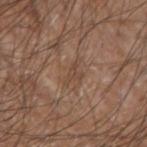Measured at roughly 3 mm in maximum diameter. From the left forearm. The total-body-photography lesion software estimated an area of roughly 3 mm², an outline eccentricity of about 0.85 (0 = round, 1 = elongated), and a symmetry-axis asymmetry near 0.6. The analysis additionally found a mean CIELAB color near L≈45 a*≈17 b*≈27, roughly 6 lightness units darker than nearby skin, and a normalized lesion–skin contrast near 5.5. The analysis additionally found a border-irregularity rating of about 7/10, a within-lesion color-variation index near 0/10, and radial color variation of about 0. The analysis additionally found a classifier nevus-likeness of about 0/100 and lesion-presence confidence of about 85/100. The tile uses white-light illumination. A 15 mm crop from a total-body photograph taken for skin-cancer surveillance. A male patient roughly 55 years of age.